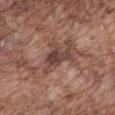follow-up: catalogued during a skin exam; not biopsied
lighting: white-light
patient: male, in their mid-70s
anatomic site: the back
image-analysis metrics: an area of roughly 4 mm² and a shape-asymmetry score of about 0.35 (0 = symmetric); an average lesion color of about L≈40 a*≈18 b*≈21 (CIELAB), roughly 12 lightness units darker than nearby skin, and a normalized border contrast of about 10; border irregularity of about 4 on a 0–10 scale and peripheral color asymmetry of about 0.5
acquisition: 15 mm crop, total-body photography